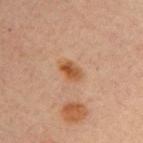follow-up=imaged on a skin check; not biopsied | patient=male, roughly 30 years of age | image=~15 mm tile from a whole-body skin photo | location=the right upper arm.